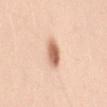Recorded during total-body skin imaging; not selected for excision or biopsy. About 3 mm across. Captured under white-light illumination. A female subject about 25 years old. A close-up tile cropped from a whole-body skin photograph, about 15 mm across. From the right upper arm.